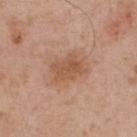Recorded during total-body skin imaging; not selected for excision or biopsy. A male patient, roughly 55 years of age. The lesion is located on the upper back. Imaged with white-light lighting. Approximately 4.5 mm at its widest. A 15 mm close-up tile from a total-body photography series done for melanoma screening.